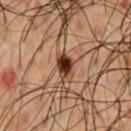Q: Was this lesion biopsied?
A: total-body-photography surveillance lesion; no biopsy
Q: Illumination type?
A: cross-polarized illumination
Q: How large is the lesion?
A: ≈3.5 mm
Q: Automated lesion metrics?
A: a lesion color around L≈27 a*≈17 b*≈24 in CIELAB, a lesion–skin lightness drop of about 14, and a normalized border contrast of about 13.5; a border-irregularity index near 2/10 and a within-lesion color-variation index near 5.5/10; an automated nevus-likeness rating near 100 out of 100 and a lesion-detection confidence of about 100/100
Q: What is the imaging modality?
A: ~15 mm crop, total-body skin-cancer survey
Q: Patient demographics?
A: male, aged around 50
Q: Lesion location?
A: the chest